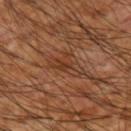Imaged during a routine full-body skin examination; the lesion was not biopsied and no histopathology is available.